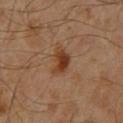Q: Was this lesion biopsied?
A: total-body-photography surveillance lesion; no biopsy
Q: What kind of image is this?
A: ~15 mm tile from a whole-body skin photo
Q: Lesion location?
A: the chest
Q: What lighting was used for the tile?
A: cross-polarized illumination
Q: Patient demographics?
A: male, aged around 60
Q: What did automated image analysis measure?
A: a mean CIELAB color near L≈35 a*≈20 b*≈30, a lesion–skin lightness drop of about 10, and a normalized lesion–skin contrast near 9.5